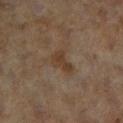  biopsy_status: not biopsied; imaged during a skin examination
  site: right lower leg
  patient:
    sex: female
    age_approx: 60
  image:
    source: total-body photography crop
    field_of_view_mm: 15